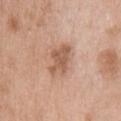This lesion was catalogued during total-body skin photography and was not selected for biopsy.
On the upper back.
A male subject, roughly 40 years of age.
Measured at roughly 4 mm in maximum diameter.
A lesion tile, about 15 mm wide, cut from a 3D total-body photograph.